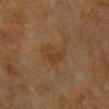notes: imaged on a skin check; not biopsied
imaging modality: total-body-photography crop, ~15 mm field of view
patient: female, aged approximately 80
image-analysis metrics: a footprint of about 5.5 mm² and an eccentricity of roughly 0.75; roughly 5 lightness units darker than nearby skin and a lesion-to-skin contrast of about 5.5 (normalized; higher = more distinct); a nevus-likeness score of about 0/100 and a lesion-detection confidence of about 100/100
body site: the upper back
illumination: cross-polarized illumination
size: ~3.5 mm (longest diameter)